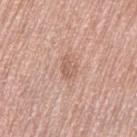No biopsy was performed on this lesion — it was imaged during a full skin examination and was not determined to be concerning. Captured under white-light illumination. A female patient aged 58–62. The lesion's longest dimension is about 2.5 mm. The lesion is on the left thigh. A region of skin cropped from a whole-body photographic capture, roughly 15 mm wide. Automated image analysis of the tile measured an average lesion color of about L≈60 a*≈20 b*≈28 (CIELAB), roughly 8 lightness units darker than nearby skin, and a lesion-to-skin contrast of about 5.5 (normalized; higher = more distinct). And it measured a nevus-likeness score of about 0/100 and lesion-presence confidence of about 100/100.